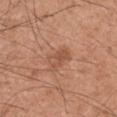notes: no biopsy performed (imaged during a skin exam) | subject: male, aged approximately 60 | image source: total-body-photography crop, ~15 mm field of view | site: the right upper arm | automated lesion analysis: an average lesion color of about L≈52 a*≈24 b*≈32 (CIELAB), roughly 8 lightness units darker than nearby skin, and a lesion-to-skin contrast of about 5.5 (normalized; higher = more distinct); a nevus-likeness score of about 40/100 | lighting: white-light illumination.